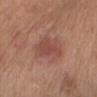follow-up — total-body-photography surveillance lesion; no biopsy
lighting — white-light
acquisition — total-body-photography crop, ~15 mm field of view
site — the chest
subject — female, roughly 55 years of age
diameter — ≈5.5 mm